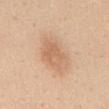{"patient": {"sex": "female", "age_approx": 45}, "lighting": "white-light", "lesion_size": {"long_diameter_mm_approx": 5.5}, "site": "abdomen", "image": {"source": "total-body photography crop", "field_of_view_mm": 15}}